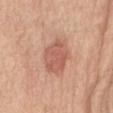No biopsy was performed on this lesion — it was imaged during a full skin examination and was not determined to be concerning.
Measured at roughly 5 mm in maximum diameter.
A roughly 15 mm field-of-view crop from a total-body skin photograph.
An algorithmic analysis of the crop reported a lesion color around L≈59 a*≈25 b*≈29 in CIELAB, a lesion–skin lightness drop of about 10, and a normalized border contrast of about 6.5. The analysis additionally found a classifier nevus-likeness of about 40/100 and a detector confidence of about 100 out of 100 that the crop contains a lesion.
A female subject about 75 years old.
From the abdomen.
This is a white-light tile.